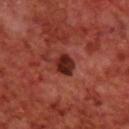No biopsy was performed on this lesion — it was imaged during a full skin examination and was not determined to be concerning. The lesion is located on the upper back. Imaged with cross-polarized lighting. An algorithmic analysis of the crop reported a lesion area of about 5.5 mm² and a symmetry-axis asymmetry near 0.2. It also reported a nevus-likeness score of about 30/100 and a detector confidence of about 100 out of 100 that the crop contains a lesion. The lesion's longest dimension is about 3 mm. A 15 mm close-up extracted from a 3D total-body photography capture. A male patient, approximately 70 years of age.